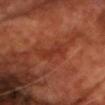Acquisition and patient details:
Cropped from a total-body skin-imaging series; the visible field is about 15 mm. A male subject, roughly 70 years of age. Imaged with cross-polarized lighting. Automated image analysis of the tile measured an average lesion color of about L≈34 a*≈30 b*≈33 (CIELAB) and a lesion–skin lightness drop of about 6. It also reported a border-irregularity rating of about 5/10, internal color variation of about 0 on a 0–10 scale, and peripheral color asymmetry of about 0. And it measured an automated nevus-likeness rating near 0 out of 100. Located on the chest.